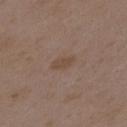workup=total-body-photography surveillance lesion; no biopsy.